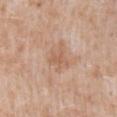notes: total-body-photography surveillance lesion; no biopsy
subject: male, aged 48–52
location: the mid back
tile lighting: white-light
imaging modality: 15 mm crop, total-body photography
lesion diameter: about 3 mm